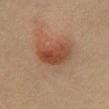Context:
The tile uses cross-polarized illumination. Automated tile analysis of the lesion measured a classifier nevus-likeness of about 100/100 and a detector confidence of about 100 out of 100 that the crop contains a lesion. The lesion is located on the chest. A region of skin cropped from a whole-body photographic capture, roughly 15 mm wide. The patient is a male aged approximately 40.